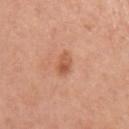Assessment:
No biopsy was performed on this lesion — it was imaged during a full skin examination and was not determined to be concerning.
Background:
Located on the left upper arm. A 15 mm close-up tile from a total-body photography series done for melanoma screening. A female patient aged 63–67.